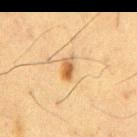– follow-up: catalogued during a skin exam; not biopsied
– site: the chest
– acquisition: ~15 mm tile from a whole-body skin photo
– patient: male, about 60 years old
– illumination: cross-polarized illumination
– size: about 2.5 mm
– automated metrics: a shape-asymmetry score of about 0.25 (0 = symmetric); border irregularity of about 2 on a 0–10 scale and a peripheral color-asymmetry measure near 1; an automated nevus-likeness rating near 95 out of 100 and lesion-presence confidence of about 100/100